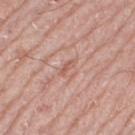Q: Is there a histopathology result?
A: catalogued during a skin exam; not biopsied
Q: How was this image acquired?
A: ~15 mm crop, total-body skin-cancer survey
Q: What are the patient's age and sex?
A: male, aged 68–72
Q: How large is the lesion?
A: ≈2.5 mm
Q: How was the tile lit?
A: white-light illumination
Q: Lesion location?
A: the right thigh